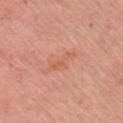| key | value |
|---|---|
| follow-up | total-body-photography surveillance lesion; no biopsy |
| patient | female, approximately 55 years of age |
| lesion diameter | ~4 mm (longest diameter) |
| site | the right upper arm |
| imaging modality | ~15 mm tile from a whole-body skin photo |
| illumination | white-light illumination |